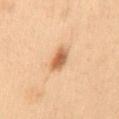Q: Is there a histopathology result?
A: catalogued during a skin exam; not biopsied
Q: Automated lesion metrics?
A: an area of roughly 5 mm², an eccentricity of roughly 0.75, and a shape-asymmetry score of about 0.35 (0 = symmetric); about 12 CIELAB-L* units darker than the surrounding skin; a nevus-likeness score of about 95/100 and a detector confidence of about 100 out of 100 that the crop contains a lesion
Q: Where on the body is the lesion?
A: the mid back
Q: Lesion size?
A: about 3 mm
Q: What are the patient's age and sex?
A: male, aged 53 to 57
Q: What kind of image is this?
A: ~15 mm crop, total-body skin-cancer survey